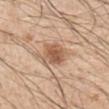Part of a total-body skin-imaging series; this lesion was reviewed on a skin check and was not flagged for biopsy. A 15 mm crop from a total-body photograph taken for skin-cancer surveillance. A male subject about 50 years old. On the right upper arm.